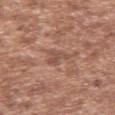This lesion was catalogued during total-body skin photography and was not selected for biopsy. A 15 mm close-up tile from a total-body photography series done for melanoma screening. The lesion is located on the left upper arm. Measured at roughly 3.5 mm in maximum diameter. A male patient aged 43 to 47. The tile uses white-light illumination.